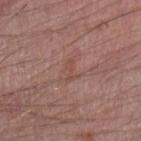Q: Was this lesion biopsied?
A: no biopsy performed (imaged during a skin exam)
Q: What is the lesion's diameter?
A: about 2.5 mm
Q: What lighting was used for the tile?
A: white-light illumination
Q: Where on the body is the lesion?
A: the left forearm
Q: What are the patient's age and sex?
A: male, roughly 50 years of age
Q: How was this image acquired?
A: total-body-photography crop, ~15 mm field of view